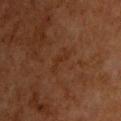No biopsy was performed on this lesion — it was imaged during a full skin examination and was not determined to be concerning.
The lesion is located on the upper back.
A 15 mm close-up extracted from a 3D total-body photography capture.
Captured under cross-polarized illumination.
Automated image analysis of the tile measured a footprint of about 3 mm² and an eccentricity of roughly 0.9. The software also gave a mean CIELAB color near L≈25 a*≈18 b*≈27, roughly 4 lightness units darker than nearby skin, and a lesion-to-skin contrast of about 4.5 (normalized; higher = more distinct).
A male subject roughly 65 years of age.
Measured at roughly 3 mm in maximum diameter.